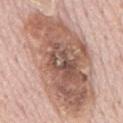Case summary:
– patient: male, about 75 years old
– TBP lesion metrics: a lesion color around L≈58 a*≈19 b*≈27 in CIELAB, a lesion–skin lightness drop of about 15, and a normalized border contrast of about 9.5; a color-variation rating of about 8.5/10 and a peripheral color-asymmetry measure near 3
– lesion size: ≈14.5 mm
– body site: the mid back
– tile lighting: white-light
– image source: ~15 mm tile from a whole-body skin photo